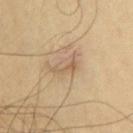Clinical impression: Recorded during total-body skin imaging; not selected for excision or biopsy. Context: The lesion is on the front of the torso. A roughly 15 mm field-of-view crop from a total-body skin photograph. A male subject, about 55 years old. The tile uses cross-polarized illumination. Measured at roughly 3.5 mm in maximum diameter. The lesion-visualizer software estimated an area of roughly 3.5 mm², an eccentricity of roughly 0.9, and a symmetry-axis asymmetry near 0.85.